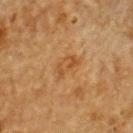Captured during whole-body skin photography for melanoma surveillance; the lesion was not biopsied. The lesion is on the upper back. A lesion tile, about 15 mm wide, cut from a 3D total-body photograph. The patient is a male aged around 85 or older.